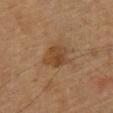Case summary:
- follow-up: imaged on a skin check; not biopsied
- illumination: cross-polarized illumination
- imaging modality: ~15 mm tile from a whole-body skin photo
- patient: male, about 60 years old
- automated metrics: a footprint of about 7.5 mm² and two-axis asymmetry of about 0.2; an automated nevus-likeness rating near 15 out of 100
- diameter: about 3.5 mm
- location: the back